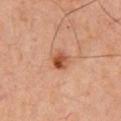Q: How was this image acquired?
A: ~15 mm tile from a whole-body skin photo
Q: Illumination type?
A: cross-polarized
Q: Automated lesion metrics?
A: a lesion area of about 4 mm², an outline eccentricity of about 0.4 (0 = round, 1 = elongated), and two-axis asymmetry of about 0.25
Q: What are the patient's age and sex?
A: aged 63 to 67
Q: Lesion location?
A: the front of the torso
Q: How large is the lesion?
A: about 2 mm